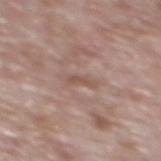The lesion was photographed on a routine skin check and not biopsied; there is no pathology result. The recorded lesion diameter is about 2.5 mm. The lesion is located on the back. The tile uses white-light illumination. A 15 mm crop from a total-body photograph taken for skin-cancer surveillance. The patient is a male in their 60s. Automated tile analysis of the lesion measured a mean CIELAB color near L≈52 a*≈18 b*≈25. The software also gave a border-irregularity index near 5.5/10, internal color variation of about 0 on a 0–10 scale, and radial color variation of about 0. And it measured a classifier nevus-likeness of about 0/100 and lesion-presence confidence of about 90/100.